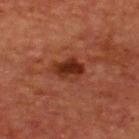Q: Is there a histopathology result?
A: total-body-photography surveillance lesion; no biopsy
Q: What is the lesion's diameter?
A: about 3.5 mm
Q: Automated lesion metrics?
A: an automated nevus-likeness rating near 80 out of 100 and a detector confidence of about 100 out of 100 that the crop contains a lesion
Q: Illumination type?
A: cross-polarized illumination
Q: Where on the body is the lesion?
A: the upper back
Q: How was this image acquired?
A: ~15 mm crop, total-body skin-cancer survey
Q: Who is the patient?
A: male, aged 63–67